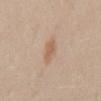Imaged during a routine full-body skin examination; the lesion was not biopsied and no histopathology is available. The patient is a female about 40 years old. Located on the mid back. Measured at roughly 4 mm in maximum diameter. A close-up tile cropped from a whole-body skin photograph, about 15 mm across. Captured under white-light illumination.